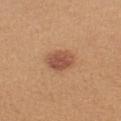Case summary:
– follow-up: total-body-photography surveillance lesion; no biopsy
– lesion size: ≈3.5 mm
– imaging modality: ~15 mm crop, total-body skin-cancer survey
– lighting: white-light
– patient: female, aged 23–27
– body site: the left upper arm
– automated metrics: an area of roughly 7.5 mm², a shape eccentricity near 0.6, and two-axis asymmetry of about 0.15; a border-irregularity rating of about 1.5/10, internal color variation of about 3 on a 0–10 scale, and radial color variation of about 1; a nevus-likeness score of about 95/100 and a detector confidence of about 100 out of 100 that the crop contains a lesion